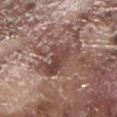| key | value |
|---|---|
| biopsy status | imaged on a skin check; not biopsied |
| site | the right forearm |
| subject | male, aged around 75 |
| acquisition | 15 mm crop, total-body photography |